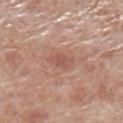This lesion was catalogued during total-body skin photography and was not selected for biopsy. Measured at roughly 3 mm in maximum diameter. A 15 mm close-up extracted from a 3D total-body photography capture. The lesion is located on the left lower leg. A male patient, approximately 70 years of age. The total-body-photography lesion software estimated a footprint of about 5.5 mm². The software also gave an automated nevus-likeness rating near 0 out of 100 and a detector confidence of about 100 out of 100 that the crop contains a lesion. The tile uses white-light illumination.